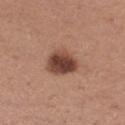Notes:
- notes: no biopsy performed (imaged during a skin exam)
- acquisition: 15 mm crop, total-body photography
- illumination: white-light
- lesion size: ≈4 mm
- body site: the right thigh
- automated lesion analysis: an area of roughly 11 mm², an outline eccentricity of about 0.5 (0 = round, 1 = elongated), and two-axis asymmetry of about 0.2; a lesion color around L≈44 a*≈22 b*≈26 in CIELAB, roughly 15 lightness units darker than nearby skin, and a normalized border contrast of about 11; border irregularity of about 2 on a 0–10 scale and peripheral color asymmetry of about 2; an automated nevus-likeness rating near 90 out of 100 and lesion-presence confidence of about 100/100
- subject: female, aged 28–32